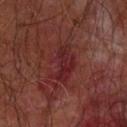No biopsy was performed on this lesion — it was imaged during a full skin examination and was not determined to be concerning.
A male patient in their mid- to late 60s.
Approximately 5 mm at its widest.
A lesion tile, about 15 mm wide, cut from a 3D total-body photograph.
Imaged with cross-polarized lighting.
On the left forearm.
Automated tile analysis of the lesion measured a footprint of about 10 mm². And it measured a border-irregularity index near 5/10, a within-lesion color-variation index near 5/10, and peripheral color asymmetry of about 1.5.